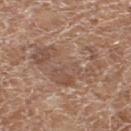Q: What did automated image analysis measure?
A: a lesion area of about 24 mm², a shape eccentricity near 0.8, and two-axis asymmetry of about 0.6; a lesion–skin lightness drop of about 7 and a lesion-to-skin contrast of about 5 (normalized; higher = more distinct); an automated nevus-likeness rating near 0 out of 100 and lesion-presence confidence of about 70/100
Q: Who is the patient?
A: female, approximately 75 years of age
Q: What lighting was used for the tile?
A: white-light illumination
Q: What is the lesion's diameter?
A: ~8 mm (longest diameter)
Q: Lesion location?
A: the left thigh
Q: How was this image acquired?
A: total-body-photography crop, ~15 mm field of view